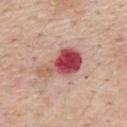Assessment: Part of a total-body skin-imaging series; this lesion was reviewed on a skin check and was not flagged for biopsy.